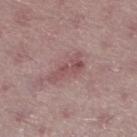Impression:
No biopsy was performed on this lesion — it was imaged during a full skin examination and was not determined to be concerning.
Image and clinical context:
A 15 mm close-up extracted from a 3D total-body photography capture. The patient is a male about 50 years old. The lesion is located on the left lower leg.